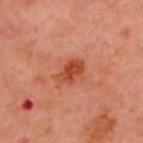<lesion>
<biopsy_status>not biopsied; imaged during a skin examination</biopsy_status>
<image>
  <source>total-body photography crop</source>
  <field_of_view_mm>15</field_of_view_mm>
</image>
<site>upper back</site>
<automated_metrics>
  <area_mm2_approx>6.5</area_mm2_approx>
  <eccentricity>0.75</eccentricity>
  <nevus_likeness_0_100>85</nevus_likeness_0_100>
  <lesion_detection_confidence_0_100>100</lesion_detection_confidence_0_100>
</automated_metrics>
<lighting>cross-polarized</lighting>
<patient>
  <sex>male</sex>
  <age_approx>50</age_approx>
</patient>
</lesion>